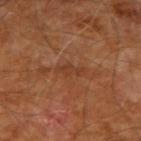Clinical impression:
The lesion was photographed on a routine skin check and not biopsied; there is no pathology result.
Image and clinical context:
The lesion-visualizer software estimated a lesion area of about 3.5 mm², an eccentricity of roughly 0.9, and a symmetry-axis asymmetry near 0.35. The analysis additionally found an average lesion color of about L≈38 a*≈23 b*≈32 (CIELAB) and a lesion-to-skin contrast of about 5 (normalized; higher = more distinct). It also reported a peripheral color-asymmetry measure near 0. The lesion's longest dimension is about 3.5 mm. A 15 mm crop from a total-body photograph taken for skin-cancer surveillance. Located on the right upper arm. The patient is a male aged approximately 70. The tile uses cross-polarized illumination.